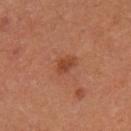Captured during whole-body skin photography for melanoma surveillance; the lesion was not biopsied.
The patient is a male approximately 60 years of age.
A close-up tile cropped from a whole-body skin photograph, about 15 mm across.
This is a cross-polarized tile.
On the leg.